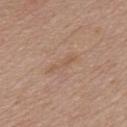{"patient": {"sex": "male", "age_approx": 55}, "site": "chest", "image": {"source": "total-body photography crop", "field_of_view_mm": 15}, "automated_metrics": {"nevus_likeness_0_100": 0, "lesion_detection_confidence_0_100": 100}, "lesion_size": {"long_diameter_mm_approx": 4.0}, "lighting": "white-light"}